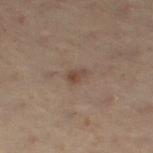Case summary:
• workup: imaged on a skin check; not biopsied
• subject: female, roughly 55 years of age
• acquisition: total-body-photography crop, ~15 mm field of view
• site: the left leg